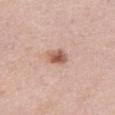Clinical impression: The lesion was photographed on a routine skin check and not biopsied; there is no pathology result. Context: From the chest. The lesion's longest dimension is about 2.5 mm. A lesion tile, about 15 mm wide, cut from a 3D total-body photograph. The subject is a female aged approximately 60.